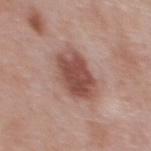biopsy_status: not biopsied; imaged during a skin examination
patient:
  sex: male
  age_approx: 55
automated_metrics:
  eccentricity: 0.7
  shape_asymmetry: 0.15
  cielab_L: 50
  cielab_a: 22
  cielab_b: 25
  vs_skin_contrast_norm: 9.0
  nevus_likeness_0_100: 90
lesion_size:
  long_diameter_mm_approx: 5.5
image:
  source: total-body photography crop
  field_of_view_mm: 15
lighting: white-light
site: chest